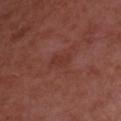follow-up=imaged on a skin check; not biopsied | tile lighting=white-light illumination | body site=the front of the torso | diameter=~2.5 mm (longest diameter) | patient=male, aged 63–67 | TBP lesion metrics=a border-irregularity index near 3/10; a classifier nevus-likeness of about 0/100 and a detector confidence of about 100 out of 100 that the crop contains a lesion | imaging modality=~15 mm tile from a whole-body skin photo.